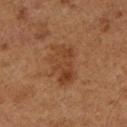Impression:
Part of a total-body skin-imaging series; this lesion was reviewed on a skin check and was not flagged for biopsy.
Acquisition and patient details:
Measured at roughly 5 mm in maximum diameter. A lesion tile, about 15 mm wide, cut from a 3D total-body photograph. A male subject in their mid- to late 70s. Imaged with cross-polarized lighting. On the left lower leg.